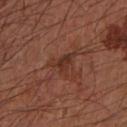notes — imaged on a skin check; not biopsied
image — total-body-photography crop, ~15 mm field of view
automated metrics — a lesion area of about 5.5 mm², an eccentricity of roughly 0.6, and a shape-asymmetry score of about 0.55 (0 = symmetric)
location — the left forearm
patient — male, in their mid- to late 60s
lesion diameter — about 3.5 mm